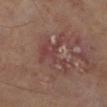The recorded lesion diameter is about 6 mm.
A 15 mm close-up tile from a total-body photography series done for melanoma screening.
From the left lower leg.
The subject is a male roughly 65 years of age.
Histopathological examination showed a squamous cell carcinoma in situ.